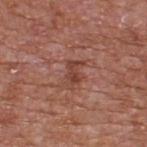follow-up: catalogued during a skin exam; not biopsied
illumination: white-light illumination
anatomic site: the upper back
image-analysis metrics: an eccentricity of roughly 0.8 and a symmetry-axis asymmetry near 0.4; a lesion color around L≈43 a*≈24 b*≈27 in CIELAB and a lesion-to-skin contrast of about 7 (normalized; higher = more distinct); a border-irregularity index near 5/10 and a color-variation rating of about 2/10
image: ~15 mm tile from a whole-body skin photo
subject: male, aged approximately 65
lesion size: ~3 mm (longest diameter)